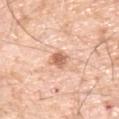Q: How large is the lesion?
A: about 3 mm
Q: Patient demographics?
A: male, in their 70s
Q: What did automated image analysis measure?
A: an area of roughly 4.5 mm², an eccentricity of roughly 0.75, and a symmetry-axis asymmetry near 0.2; a mean CIELAB color near L≈66 a*≈24 b*≈33, roughly 13 lightness units darker than nearby skin, and a lesion-to-skin contrast of about 8 (normalized; higher = more distinct); a border-irregularity index near 2.5/10, a color-variation rating of about 3/10, and a peripheral color-asymmetry measure near 1
Q: What lighting was used for the tile?
A: white-light illumination
Q: Lesion location?
A: the front of the torso
Q: What is the imaging modality?
A: ~15 mm tile from a whole-body skin photo